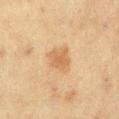Impression:
The lesion was tiled from a total-body skin photograph and was not biopsied.
Background:
This image is a 15 mm lesion crop taken from a total-body photograph. The lesion is located on the leg. A female subject in their 50s.